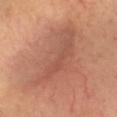biopsy status: imaged on a skin check; not biopsied | image source: 15 mm crop, total-body photography | subject: male, aged approximately 55 | size: ~11 mm (longest diameter) | lighting: cross-polarized | anatomic site: the head or neck | TBP lesion metrics: a footprint of about 37 mm², a shape eccentricity near 0.9, and two-axis asymmetry of about 0.4; a border-irregularity rating of about 6/10, internal color variation of about 4.5 on a 0–10 scale, and radial color variation of about 1.5; a nevus-likeness score of about 35/100 and a detector confidence of about 55 out of 100 that the crop contains a lesion.